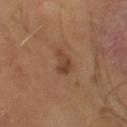notes=total-body-photography surveillance lesion; no biopsy | patient=male, in their mid- to late 60s | tile lighting=cross-polarized | imaging modality=~15 mm crop, total-body skin-cancer survey | diameter=about 3 mm.